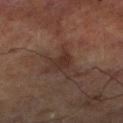Q: Who is the patient?
A: male, aged around 70
Q: What is the imaging modality?
A: ~15 mm tile from a whole-body skin photo
Q: How large is the lesion?
A: ≈3 mm
Q: What is the anatomic site?
A: the leg